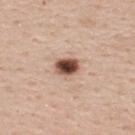Assessment:
This lesion was catalogued during total-body skin photography and was not selected for biopsy.
Context:
Located on the upper back. A female subject in their mid- to late 50s. Automated image analysis of the tile measured a lesion area of about 5.5 mm² and an eccentricity of roughly 0.7. The analysis additionally found a mean CIELAB color near L≈49 a*≈21 b*≈26, a lesion–skin lightness drop of about 22, and a lesion-to-skin contrast of about 14 (normalized; higher = more distinct). It also reported border irregularity of about 1.5 on a 0–10 scale, a color-variation rating of about 7/10, and peripheral color asymmetry of about 2. It also reported an automated nevus-likeness rating near 100 out of 100 and a detector confidence of about 100 out of 100 that the crop contains a lesion. Cropped from a whole-body photographic skin survey; the tile spans about 15 mm. About 3 mm across.